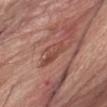Notes:
- workup: catalogued during a skin exam; not biopsied
- illumination: white-light illumination
- anatomic site: the abdomen
- image source: ~15 mm crop, total-body skin-cancer survey
- subject: female, aged 73 to 77
- diameter: about 4.5 mm